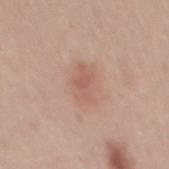biopsy status: imaged on a skin check; not biopsied
lesion diameter: ~4 mm (longest diameter)
patient: male, about 45 years old
lighting: white-light illumination
location: the mid back
image source: 15 mm crop, total-body photography